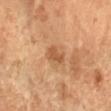This lesion was catalogued during total-body skin photography and was not selected for biopsy. A 15 mm crop from a total-body photograph taken for skin-cancer surveillance. The patient is a female aged 68–72. On the right forearm.